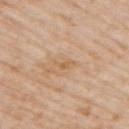Case summary:
- notes — no biopsy performed (imaged during a skin exam)
- body site — the right upper arm
- lighting — white-light illumination
- acquisition — total-body-photography crop, ~15 mm field of view
- subject — male, aged approximately 75
- diameter — ≈2.5 mm